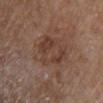| feature | finding |
|---|---|
| notes | no biopsy performed (imaged during a skin exam) |
| tile lighting | white-light |
| patient | female, roughly 85 years of age or older |
| image source | ~15 mm crop, total-body skin-cancer survey |
| size | ≈4.5 mm |
| site | the front of the torso |